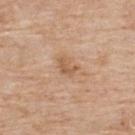- subject: female, about 65 years old
- site: the upper back
- automated metrics: a lesion area of about 4 mm², an outline eccentricity of about 0.7 (0 = round, 1 = elongated), and a shape-asymmetry score of about 0.35 (0 = symmetric); border irregularity of about 3.5 on a 0–10 scale and peripheral color asymmetry of about 0.5
- image source: 15 mm crop, total-body photography
- diameter: ≈2.5 mm